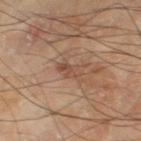The subject is a male approximately 65 years of age. A close-up tile cropped from a whole-body skin photograph, about 15 mm across. On the right thigh.